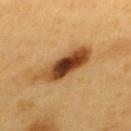Clinical impression: Recorded during total-body skin imaging; not selected for excision or biopsy. Acquisition and patient details: A male patient aged approximately 60. A close-up tile cropped from a whole-body skin photograph, about 15 mm across. The total-body-photography lesion software estimated a lesion area of about 15 mm², a shape eccentricity near 0.95, and a shape-asymmetry score of about 0.25 (0 = symmetric). And it measured an average lesion color of about L≈46 a*≈22 b*≈39 (CIELAB), roughly 18 lightness units darker than nearby skin, and a lesion-to-skin contrast of about 13 (normalized; higher = more distinct). The analysis additionally found border irregularity of about 3.5 on a 0–10 scale and a within-lesion color-variation index near 9/10. The lesion is located on the upper back. The lesion's longest dimension is about 7.5 mm. This is a cross-polarized tile.